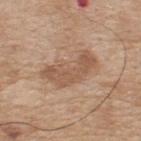Recorded during total-body skin imaging; not selected for excision or biopsy.
Automated tile analysis of the lesion measured a footprint of about 14 mm² and an outline eccentricity of about 0.9 (0 = round, 1 = elongated). The software also gave an average lesion color of about L≈55 a*≈18 b*≈31 (CIELAB), about 9 CIELAB-L* units darker than the surrounding skin, and a lesion-to-skin contrast of about 6.5 (normalized; higher = more distinct).
Cropped from a total-body skin-imaging series; the visible field is about 15 mm.
From the upper back.
A male subject aged 73 to 77.
Longest diameter approximately 6 mm.